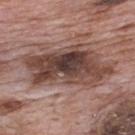Findings:
* biopsy status · no biopsy performed (imaged during a skin exam)
* subject · male, aged 68–72
* site · the upper back
* image · total-body-photography crop, ~15 mm field of view
* size · about 10.5 mm
* illumination · white-light illumination